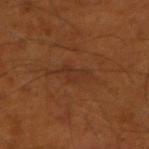follow-up = imaged on a skin check; not biopsied.